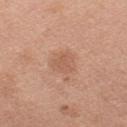Part of a total-body skin-imaging series; this lesion was reviewed on a skin check and was not flagged for biopsy. Located on the left upper arm. This is a white-light tile. Cropped from a whole-body photographic skin survey; the tile spans about 15 mm. Approximately 2.5 mm at its widest. The subject is a female aged around 50.